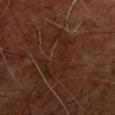Assessment:
This lesion was catalogued during total-body skin photography and was not selected for biopsy.
Clinical summary:
Cropped from a whole-body photographic skin survey; the tile spans about 15 mm. From the upper back. The recorded lesion diameter is about 7 mm. A male patient about 55 years old.